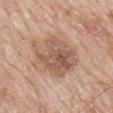follow-up — total-body-photography surveillance lesion; no biopsy | size — ~6.5 mm (longest diameter) | automated metrics — an area of roughly 22 mm² and a shape eccentricity near 0.65; an automated nevus-likeness rating near 10 out of 100 and a lesion-detection confidence of about 100/100 | illumination — white-light | image source — ~15 mm crop, total-body skin-cancer survey | site — the back | patient — male, aged 63 to 67.